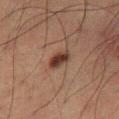Imaged during a routine full-body skin examination; the lesion was not biopsied and no histopathology is available.
Measured at roughly 3 mm in maximum diameter.
A 15 mm crop from a total-body photograph taken for skin-cancer surveillance.
The subject is a male aged around 60.
The tile uses cross-polarized illumination.